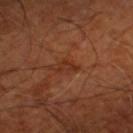Context: A patient in their mid- to late 60s. A close-up tile cropped from a whole-body skin photograph, about 15 mm across. Located on the left thigh. Captured under cross-polarized illumination. An algorithmic analysis of the crop reported a footprint of about 3 mm² and an outline eccentricity of about 0.8 (0 = round, 1 = elongated).